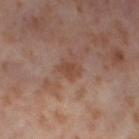follow-up: no biopsy performed (imaged during a skin exam) | body site: the left thigh | imaging modality: total-body-photography crop, ~15 mm field of view | automated metrics: a shape eccentricity near 0.7 and a shape-asymmetry score of about 0.2 (0 = symmetric); a mean CIELAB color near L≈46 a*≈21 b*≈28, a lesion–skin lightness drop of about 7, and a lesion-to-skin contrast of about 6.5 (normalized; higher = more distinct); a border-irregularity index near 1.5/10 and a peripheral color-asymmetry measure near 0.5 | size: ≈2.5 mm | subject: female, roughly 55 years of age.